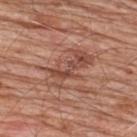| key | value |
|---|---|
| workup | imaged on a skin check; not biopsied |
| lesion size | ≈6 mm |
| location | the back |
| imaging modality | ~15 mm crop, total-body skin-cancer survey |
| subject | male, in their 80s |